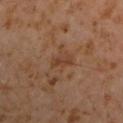Q: Was a biopsy performed?
A: no biopsy performed (imaged during a skin exam)
Q: What did automated image analysis measure?
A: a footprint of about 3 mm², an eccentricity of roughly 0.9, and a symmetry-axis asymmetry near 0.4; a mean CIELAB color near L≈36 a*≈19 b*≈29 and a lesion–skin lightness drop of about 6; a border-irregularity index near 4.5/10, a color-variation rating of about 0/10, and peripheral color asymmetry of about 0; a lesion-detection confidence of about 100/100
Q: What is the anatomic site?
A: the left upper arm
Q: Lesion size?
A: about 2.5 mm
Q: How was this image acquired?
A: ~15 mm crop, total-body skin-cancer survey
Q: What are the patient's age and sex?
A: male, in their 60s